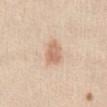{
  "biopsy_status": "not biopsied; imaged during a skin examination",
  "image": {
    "source": "total-body photography crop",
    "field_of_view_mm": 15
  },
  "patient": {
    "sex": "female",
    "age_approx": 40
  },
  "lesion_size": {
    "long_diameter_mm_approx": 3.0
  },
  "site": "abdomen"
}